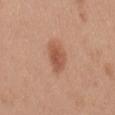| key | value |
|---|---|
| notes | imaged on a skin check; not biopsied |
| location | the mid back |
| automated lesion analysis | an average lesion color of about L≈55 a*≈24 b*≈32 (CIELAB) and a lesion–skin lightness drop of about 10 |
| diameter | about 4 mm |
| imaging modality | total-body-photography crop, ~15 mm field of view |
| subject | female, approximately 40 years of age |